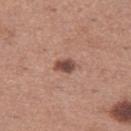This is a white-light tile. The subject is a female aged around 30. Longest diameter approximately 2.5 mm. An algorithmic analysis of the crop reported a lesion color around L≈47 a*≈21 b*≈25 in CIELAB and a lesion–skin lightness drop of about 14. Located on the left thigh. A 15 mm close-up extracted from a 3D total-body photography capture.